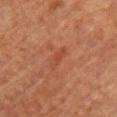follow-up = catalogued during a skin exam; not biopsied.